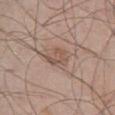notes=total-body-photography surveillance lesion; no biopsy
site=the front of the torso
subject=male, roughly 55 years of age
acquisition=~15 mm crop, total-body skin-cancer survey